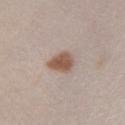Impression: Captured during whole-body skin photography for melanoma surveillance; the lesion was not biopsied. Clinical summary: Imaged with white-light lighting. The patient is a female in their mid-30s. On the right lower leg. Automated image analysis of the tile measured an average lesion color of about L≈54 a*≈17 b*≈26 (CIELAB), a lesion–skin lightness drop of about 13, and a lesion-to-skin contrast of about 9.5 (normalized; higher = more distinct). The software also gave an automated nevus-likeness rating near 100 out of 100. Cropped from a whole-body photographic skin survey; the tile spans about 15 mm.